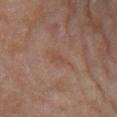workup: no biopsy performed (imaged during a skin exam) | lesion size: about 3.5 mm | site: the right lower leg | subject: female, in their 60s | acquisition: total-body-photography crop, ~15 mm field of view | automated lesion analysis: an outline eccentricity of about 0.85 (0 = round, 1 = elongated); a lesion color around L≈43 a*≈16 b*≈26 in CIELAB, a lesion–skin lightness drop of about 4, and a lesion-to-skin contrast of about 4.5 (normalized; higher = more distinct); a classifier nevus-likeness of about 0/100 and a detector confidence of about 100 out of 100 that the crop contains a lesion | lighting: cross-polarized illumination.